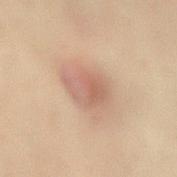Image and clinical context: This image is a 15 mm lesion crop taken from a total-body photograph. The patient is a male aged 58–62. From the mid back. Automated tile analysis of the lesion measured a lesion area of about 11 mm², an outline eccentricity of about 0.65 (0 = round, 1 = elongated), and two-axis asymmetry of about 0.2. It also reported roughly 8 lightness units darker than nearby skin and a normalized border contrast of about 5.5. It also reported a border-irregularity index near 2.5/10, a within-lesion color-variation index near 4.5/10, and radial color variation of about 1.5. Imaged with cross-polarized lighting. Approximately 4.5 mm at its widest.